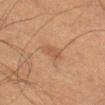A lesion tile, about 15 mm wide, cut from a 3D total-body photograph. The recorded lesion diameter is about 3 mm. The tile uses cross-polarized illumination. The lesion is on the left thigh. A male patient about 75 years old.